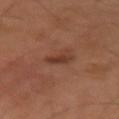Q: Is there a histopathology result?
A: total-body-photography surveillance lesion; no biopsy
Q: How was the tile lit?
A: cross-polarized
Q: What kind of image is this?
A: 15 mm crop, total-body photography
Q: How large is the lesion?
A: ~2.5 mm (longest diameter)
Q: Lesion location?
A: the right upper arm
Q: What are the patient's age and sex?
A: female, about 55 years old